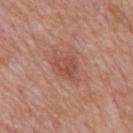Q: Was a biopsy performed?
A: total-body-photography surveillance lesion; no biopsy
Q: Where on the body is the lesion?
A: the back
Q: How was the tile lit?
A: white-light
Q: Patient demographics?
A: male, about 65 years old
Q: How was this image acquired?
A: total-body-photography crop, ~15 mm field of view